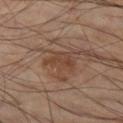biopsy status: no biopsy performed (imaged during a skin exam) | illumination: cross-polarized illumination | lesion diameter: ~3.5 mm (longest diameter) | image: total-body-photography crop, ~15 mm field of view | body site: the left thigh | patient: male, roughly 60 years of age.